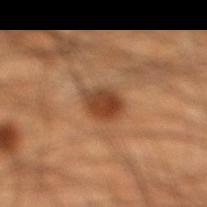| field | value |
|---|---|
| notes | total-body-photography surveillance lesion; no biopsy |
| tile lighting | cross-polarized illumination |
| TBP lesion metrics | an eccentricity of roughly 0.6 and a shape-asymmetry score of about 0.25 (0 = symmetric); a border-irregularity rating of about 2/10, internal color variation of about 4.5 on a 0–10 scale, and a peripheral color-asymmetry measure near 1.5; a nevus-likeness score of about 90/100 and lesion-presence confidence of about 100/100 |
| body site | the right lower leg |
| subject | male, aged 43–47 |
| image source | ~15 mm tile from a whole-body skin photo |